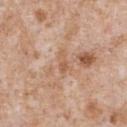Clinical impression:
The lesion was tiled from a total-body skin photograph and was not biopsied.
Clinical summary:
Approximately 2.5 mm at its widest. A male subject about 65 years old. The lesion is on the front of the torso. A roughly 15 mm field-of-view crop from a total-body skin photograph. This is a white-light tile. Automated tile analysis of the lesion measured an eccentricity of roughly 0.9 and a symmetry-axis asymmetry near 0.45. And it measured an automated nevus-likeness rating near 0 out of 100 and lesion-presence confidence of about 95/100.